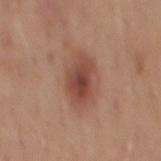No biopsy was performed on this lesion — it was imaged during a full skin examination and was not determined to be concerning. Located on the back. The tile uses white-light illumination. A male subject, roughly 50 years of age. A 15 mm crop from a total-body photograph taken for skin-cancer surveillance. The recorded lesion diameter is about 5 mm.